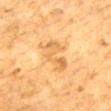<case>
<biopsy_status>not biopsied; imaged during a skin examination</biopsy_status>
<patient>
  <sex>male</sex>
  <age_approx>85+</age_approx>
</patient>
<site>mid back</site>
<lighting>cross-polarized</lighting>
<lesion_size>
  <long_diameter_mm_approx>5.0</long_diameter_mm_approx>
</lesion_size>
<automated_metrics>
  <cielab_L>60</cielab_L>
  <cielab_a>20</cielab_a>
  <cielab_b>42</cielab_b>
  <vs_skin_darker_L>8.0</vs_skin_darker_L>
  <vs_skin_contrast_norm>5.5</vs_skin_contrast_norm>
  <border_irregularity_0_10>6.5</border_irregularity_0_10>
  <color_variation_0_10>4.0</color_variation_0_10>
  <peripheral_color_asymmetry>1.5</peripheral_color_asymmetry>
  <nevus_likeness_0_100>0</nevus_likeness_0_100>
</automated_metrics>
<image>
  <source>total-body photography crop</source>
  <field_of_view_mm>15</field_of_view_mm>
</image>
</case>